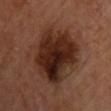The lesion was tiled from a total-body skin photograph and was not biopsied. Approximately 8 mm at its widest. On the chest. The patient is a male aged 58–62. The tile uses cross-polarized illumination. A region of skin cropped from a whole-body photographic capture, roughly 15 mm wide. An algorithmic analysis of the crop reported roughly 13 lightness units darker than nearby skin and a normalized lesion–skin contrast near 12.5. The analysis additionally found border irregularity of about 3 on a 0–10 scale, a color-variation rating of about 7.5/10, and a peripheral color-asymmetry measure near 2.5.